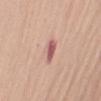Findings:
– notes · catalogued during a skin exam; not biopsied